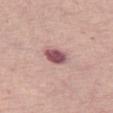biopsy status — total-body-photography surveillance lesion; no biopsy
acquisition — ~15 mm crop, total-body skin-cancer survey
lighting — white-light illumination
subject — female, approximately 65 years of age
site — the left thigh